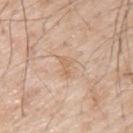Captured during whole-body skin photography for melanoma surveillance; the lesion was not biopsied. A male subject, aged around 65. Located on the left upper arm. A 15 mm close-up extracted from a 3D total-body photography capture.